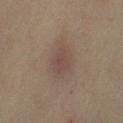Clinical impression: This lesion was catalogued during total-body skin photography and was not selected for biopsy. Background: On the right upper arm. A 15 mm close-up tile from a total-body photography series done for melanoma screening. Captured under cross-polarized illumination. The subject is a male aged around 50.